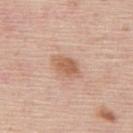Assessment: Part of a total-body skin-imaging series; this lesion was reviewed on a skin check and was not flagged for biopsy. Context: A close-up tile cropped from a whole-body skin photograph, about 15 mm across. The tile uses white-light illumination. A male patient, aged approximately 45. The recorded lesion diameter is about 3.5 mm. Located on the upper back.